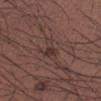follow-up: total-body-photography surveillance lesion; no biopsy
subject: male, roughly 40 years of age
acquisition: ~15 mm tile from a whole-body skin photo
body site: the right forearm
TBP lesion metrics: an area of roughly 5 mm², a shape eccentricity near 0.95, and two-axis asymmetry of about 0.5; an automated nevus-likeness rating near 5 out of 100 and a lesion-detection confidence of about 85/100